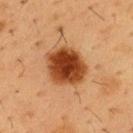notes: total-body-photography surveillance lesion; no biopsy
diameter: ≈5 mm
patient: male, aged 53–57
automated lesion analysis: an outline eccentricity of about 0.5 (0 = round, 1 = elongated) and a shape-asymmetry score of about 0.15 (0 = symmetric); about 17 CIELAB-L* units darker than the surrounding skin and a lesion-to-skin contrast of about 13.5 (normalized; higher = more distinct); a border-irregularity index near 1.5/10, a color-variation rating of about 5/10, and radial color variation of about 1.5; a nevus-likeness score of about 100/100 and a detector confidence of about 100 out of 100 that the crop contains a lesion
illumination: cross-polarized illumination
body site: the chest
image: 15 mm crop, total-body photography